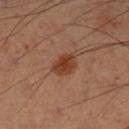From the left lower leg.
A male patient, aged around 55.
Imaged with cross-polarized lighting.
A region of skin cropped from a whole-body photographic capture, roughly 15 mm wide.
The lesion's longest dimension is about 3 mm.